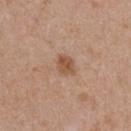This is a white-light tile.
Cropped from a total-body skin-imaging series; the visible field is about 15 mm.
Located on the chest.
A female patient, in their mid-30s.
The lesion-visualizer software estimated a shape eccentricity near 0.65 and two-axis asymmetry of about 0.3.
Measured at roughly 2.5 mm in maximum diameter.